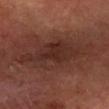Recorded during total-body skin imaging; not selected for excision or biopsy.
The lesion is located on the right forearm.
A region of skin cropped from a whole-body photographic capture, roughly 15 mm wide.
A male subject, in their 60s.